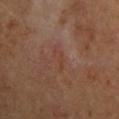Q: Was this lesion biopsied?
A: no biopsy performed (imaged during a skin exam)
Q: What kind of image is this?
A: total-body-photography crop, ~15 mm field of view
Q: What is the lesion's diameter?
A: about 2.5 mm
Q: What is the anatomic site?
A: the left upper arm
Q: What lighting was used for the tile?
A: cross-polarized illumination
Q: What did automated image analysis measure?
A: a lesion area of about 2.5 mm², an eccentricity of roughly 0.9, and two-axis asymmetry of about 0.5; border irregularity of about 5.5 on a 0–10 scale and a within-lesion color-variation index near 0/10
Q: What are the patient's age and sex?
A: male, roughly 65 years of age